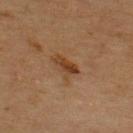notes: total-body-photography surveillance lesion; no biopsy
lesion size: ≈3.5 mm
tile lighting: cross-polarized illumination
imaging modality: ~15 mm crop, total-body skin-cancer survey
anatomic site: the upper back
automated metrics: a lesion area of about 4.5 mm², a shape eccentricity near 0.85, and a symmetry-axis asymmetry near 0.4
patient: male, approximately 65 years of age